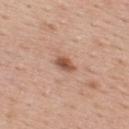workup: total-body-photography surveillance lesion; no biopsy | site: the upper back | patient: female, aged 38 to 42 | imaging modality: ~15 mm crop, total-body skin-cancer survey | lesion size: ~2.5 mm (longest diameter) | tile lighting: white-light.